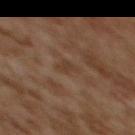Part of a total-body skin-imaging series; this lesion was reviewed on a skin check and was not flagged for biopsy.
A female patient, roughly 60 years of age.
Captured under cross-polarized illumination.
Located on the upper back.
A close-up tile cropped from a whole-body skin photograph, about 15 mm across.